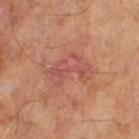This lesion was catalogued during total-body skin photography and was not selected for biopsy.
Imaged with cross-polarized lighting.
Cropped from a total-body skin-imaging series; the visible field is about 15 mm.
The patient is a male aged approximately 65.
Longest diameter approximately 5.5 mm.
Automated image analysis of the tile measured about 6 CIELAB-L* units darker than the surrounding skin and a lesion-to-skin contrast of about 5.5 (normalized; higher = more distinct). And it measured border irregularity of about 6 on a 0–10 scale and peripheral color asymmetry of about 1.5.